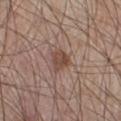Captured during whole-body skin photography for melanoma surveillance; the lesion was not biopsied. The patient is a male about 60 years old. Located on the right lower leg. Cropped from a whole-body photographic skin survey; the tile spans about 15 mm. Automated tile analysis of the lesion measured a shape eccentricity near 0.3 and a shape-asymmetry score of about 0.3 (0 = symmetric). And it measured a lesion color around L≈46 a*≈18 b*≈25 in CIELAB, roughly 10 lightness units darker than nearby skin, and a normalized lesion–skin contrast near 7.5. The recorded lesion diameter is about 2.5 mm. Imaged with white-light lighting.